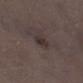Notes:
* follow-up — catalogued during a skin exam; not biopsied
* size — ~3 mm (longest diameter)
* subject — male, aged 68–72
* imaging modality — total-body-photography crop, ~15 mm field of view
* body site — the left lower leg
* tile lighting — white-light
* automated lesion analysis — a lesion area of about 3.5 mm² and a shape eccentricity near 0.85; a border-irregularity index near 2.5/10, a within-lesion color-variation index near 2/10, and radial color variation of about 0.5; an automated nevus-likeness rating near 5 out of 100 and lesion-presence confidence of about 100/100